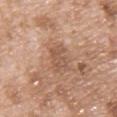Q: What did automated image analysis measure?
A: an average lesion color of about L≈54 a*≈19 b*≈30 (CIELAB) and a normalized border contrast of about 5.5; a border-irregularity rating of about 4/10, a within-lesion color-variation index near 2.5/10, and radial color variation of about 1; a nevus-likeness score of about 0/100
Q: Who is the patient?
A: male, aged approximately 50
Q: What is the lesion's diameter?
A: ≈3 mm
Q: What is the imaging modality?
A: 15 mm crop, total-body photography
Q: Where on the body is the lesion?
A: the chest
Q: How was the tile lit?
A: white-light